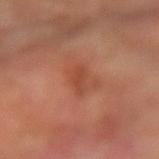Acquisition and patient details: A male subject aged 48–52. Approximately 3 mm at its widest. The lesion is located on the left arm. Captured under cross-polarized illumination. Automated image analysis of the tile measured an area of roughly 3 mm², a shape eccentricity near 0.9, and a shape-asymmetry score of about 0.35 (0 = symmetric). And it measured a mean CIELAB color near L≈46 a*≈28 b*≈33, about 7 CIELAB-L* units darker than the surrounding skin, and a normalized border contrast of about 6. The analysis additionally found a border-irregularity index near 3.5/10, internal color variation of about 1 on a 0–10 scale, and a peripheral color-asymmetry measure near 0. This image is a 15 mm lesion crop taken from a total-body photograph.